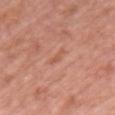{
  "biopsy_status": "not biopsied; imaged during a skin examination",
  "automated_metrics": {
    "eccentricity": 0.95,
    "shape_asymmetry": 0.45,
    "cielab_L": 56,
    "cielab_a": 25,
    "cielab_b": 33,
    "vs_skin_darker_L": 6.0,
    "vs_skin_contrast_norm": 5.0,
    "border_irregularity_0_10": 5.0,
    "color_variation_0_10": 0.0,
    "peripheral_color_asymmetry": 0.0
  },
  "site": "chest",
  "image": {
    "source": "total-body photography crop",
    "field_of_view_mm": 15
  },
  "lesion_size": {
    "long_diameter_mm_approx": 2.5
  },
  "patient": {
    "sex": "female",
    "age_approx": 40
  }
}